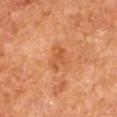biopsy status: catalogued during a skin exam; not biopsied
anatomic site: the left upper arm
automated metrics: an area of roughly 5 mm², a shape eccentricity near 0.8, and a symmetry-axis asymmetry near 0.5; a nevus-likeness score of about 0/100 and a lesion-detection confidence of about 100/100
acquisition: 15 mm crop, total-body photography
lighting: cross-polarized illumination
lesion diameter: ~3.5 mm (longest diameter)
subject: male, in their mid- to late 60s